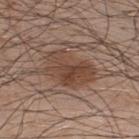  biopsy_status: not biopsied; imaged during a skin examination
  lighting: white-light
  automated_metrics:
    nevus_likeness_0_100: 90
    lesion_detection_confidence_0_100: 100
  patient:
    sex: male
    age_approx: 50
  lesion_size:
    long_diameter_mm_approx: 6.0
  site: upper back
  image:
    source: total-body photography crop
    field_of_view_mm: 15